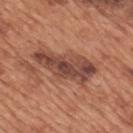  biopsy_status: not biopsied; imaged during a skin examination
  image:
    source: total-body photography crop
    field_of_view_mm: 15
  patient:
    sex: male
    age_approx: 65
  site: mid back